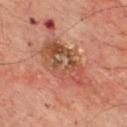Captured during whole-body skin photography for melanoma surveillance; the lesion was not biopsied.
Approximately 8 mm at its widest.
Located on the chest.
A 15 mm close-up extracted from a 3D total-body photography capture.
This is a cross-polarized tile.
An algorithmic analysis of the crop reported an eccentricity of roughly 0.9 and a shape-asymmetry score of about 0.3 (0 = symmetric). It also reported a border-irregularity index near 3.5/10, internal color variation of about 10 on a 0–10 scale, and peripheral color asymmetry of about 3.5. The software also gave an automated nevus-likeness rating near 0 out of 100 and lesion-presence confidence of about 100/100.
A male patient, aged 63–67.